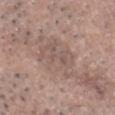size: ~6 mm (longest diameter) | patient: male, aged 68–72 | imaging modality: ~15 mm tile from a whole-body skin photo | automated lesion analysis: an eccentricity of roughly 0.8 and two-axis asymmetry of about 0.4 | site: the head or neck | lighting: white-light illumination.